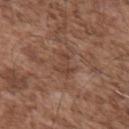workup: catalogued during a skin exam; not biopsied
diameter: about 3 mm
patient: male, about 55 years old
automated metrics: a border-irregularity index near 7.5/10, a color-variation rating of about 0.5/10, and a peripheral color-asymmetry measure near 0; a nevus-likeness score of about 0/100 and a lesion-detection confidence of about 95/100
illumination: white-light illumination
site: the upper back
acquisition: ~15 mm tile from a whole-body skin photo